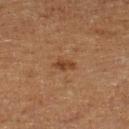No biopsy was performed on this lesion — it was imaged during a full skin examination and was not determined to be concerning. Captured under cross-polarized illumination. The lesion is located on the left lower leg. A male subject about 75 years old. Approximately 2.5 mm at its widest. Cropped from a whole-body photographic skin survey; the tile spans about 15 mm.